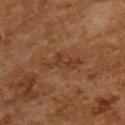Assessment: The lesion was photographed on a routine skin check and not biopsied; there is no pathology result. Acquisition and patient details: On the upper back. A female patient, roughly 60 years of age. Captured under cross-polarized illumination. Automated tile analysis of the lesion measured an eccentricity of roughly 0.9 and a symmetry-axis asymmetry near 0.4. The software also gave a border-irregularity index near 6/10, a within-lesion color-variation index near 2/10, and radial color variation of about 0.5. The recorded lesion diameter is about 5 mm. A roughly 15 mm field-of-view crop from a total-body skin photograph.